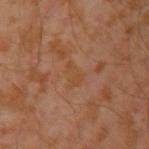Q: Was a biopsy performed?
A: no biopsy performed (imaged during a skin exam)
Q: What kind of image is this?
A: 15 mm crop, total-body photography
Q: What did automated image analysis measure?
A: a lesion area of about 3.5 mm² and an eccentricity of roughly 0.8; a border-irregularity rating of about 3.5/10, a within-lesion color-variation index near 1/10, and radial color variation of about 0.5
Q: Patient demographics?
A: male, roughly 30 years of age
Q: Lesion location?
A: the left upper arm
Q: How large is the lesion?
A: ~2.5 mm (longest diameter)